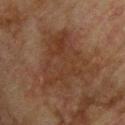Captured during whole-body skin photography for melanoma surveillance; the lesion was not biopsied.
A male subject roughly 75 years of age.
About 8 mm across.
A 15 mm close-up extracted from a 3D total-body photography capture.
The lesion is on the upper back.
The total-body-photography lesion software estimated a peripheral color-asymmetry measure near 1. The software also gave an automated nevus-likeness rating near 0 out of 100 and a detector confidence of about 100 out of 100 that the crop contains a lesion.
Imaged with cross-polarized lighting.